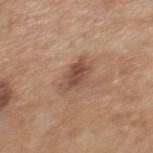Part of a total-body skin-imaging series; this lesion was reviewed on a skin check and was not flagged for biopsy. The lesion's longest dimension is about 4 mm. A roughly 15 mm field-of-view crop from a total-body skin photograph. A female patient, approximately 40 years of age. This is a white-light tile. Located on the mid back.